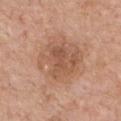The lesion was tiled from a total-body skin photograph and was not biopsied.
A roughly 15 mm field-of-view crop from a total-body skin photograph.
On the chest.
Captured under white-light illumination.
A male patient aged approximately 60.
Longest diameter approximately 6.5 mm.